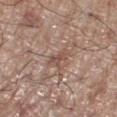Captured during whole-body skin photography for melanoma surveillance; the lesion was not biopsied.
Automated image analysis of the tile measured an area of roughly 3 mm², an outline eccentricity of about 0.75 (0 = round, 1 = elongated), and a shape-asymmetry score of about 0.4 (0 = symmetric).
This is a white-light tile.
The lesion's longest dimension is about 2.5 mm.
A 15 mm close-up extracted from a 3D total-body photography capture.
The subject is a male roughly 70 years of age.
The lesion is located on the right lower leg.